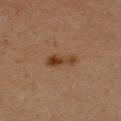The lesion was photographed on a routine skin check and not biopsied; there is no pathology result. From the left upper arm. The tile uses cross-polarized illumination. A female patient, about 40 years old. A 15 mm close-up extracted from a 3D total-body photography capture. The lesion's longest dimension is about 4 mm. The total-body-photography lesion software estimated a border-irregularity index near 4/10, a within-lesion color-variation index near 2.5/10, and radial color variation of about 0.5. The software also gave a classifier nevus-likeness of about 95/100 and a detector confidence of about 100 out of 100 that the crop contains a lesion.